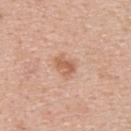The lesion was tiled from a total-body skin photograph and was not biopsied. About 3 mm across. The lesion-visualizer software estimated a lesion area of about 4.5 mm², an outline eccentricity of about 0.75 (0 = round, 1 = elongated), and two-axis asymmetry of about 0.2. The analysis additionally found a lesion color around L≈60 a*≈23 b*≈32 in CIELAB and roughly 10 lightness units darker than nearby skin. It also reported peripheral color asymmetry of about 1. This image is a 15 mm lesion crop taken from a total-body photograph. A male subject, aged 38–42. On the upper back. Captured under white-light illumination.